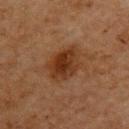{
  "biopsy_status": "not biopsied; imaged during a skin examination",
  "automated_metrics": {
    "cielab_L": 28,
    "cielab_a": 18,
    "cielab_b": 27,
    "vs_skin_darker_L": 8.0,
    "vs_skin_contrast_norm": 9.0
  },
  "patient": {
    "sex": "female",
    "age_approx": 60
  },
  "site": "upper back",
  "lighting": "cross-polarized",
  "image": {
    "source": "total-body photography crop",
    "field_of_view_mm": 15
  }
}